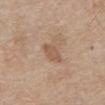follow-up: no biopsy performed (imaged during a skin exam)
size: ~3 mm (longest diameter)
body site: the abdomen
tile lighting: white-light illumination
acquisition: ~15 mm tile from a whole-body skin photo
subject: male, approximately 80 years of age